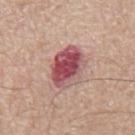workup = catalogued during a skin exam; not biopsied.